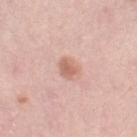Recorded during total-body skin imaging; not selected for excision or biopsy.
On the right thigh.
A lesion tile, about 15 mm wide, cut from a 3D total-body photograph.
Approximately 2.5 mm at its widest.
A female patient roughly 65 years of age.
Automated image analysis of the tile measured a lesion color around L≈64 a*≈23 b*≈28 in CIELAB, roughly 10 lightness units darker than nearby skin, and a normalized border contrast of about 6.5. And it measured a border-irregularity rating of about 2/10 and internal color variation of about 2 on a 0–10 scale. The software also gave a classifier nevus-likeness of about 65/100 and lesion-presence confidence of about 100/100.
Imaged with white-light lighting.